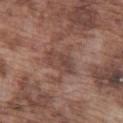biopsy_status: not biopsied; imaged during a skin examination